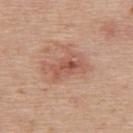No biopsy was performed on this lesion — it was imaged during a full skin examination and was not determined to be concerning.
The patient is a male aged 58–62.
The lesion's longest dimension is about 5 mm.
The tile uses white-light illumination.
This image is a 15 mm lesion crop taken from a total-body photograph.
An algorithmic analysis of the crop reported roughly 9 lightness units darker than nearby skin. And it measured a border-irregularity index near 5.5/10, a within-lesion color-variation index near 6/10, and radial color variation of about 2.
Located on the back.